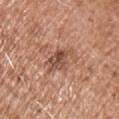Part of a total-body skin-imaging series; this lesion was reviewed on a skin check and was not flagged for biopsy.
About 4.5 mm across.
From the chest.
A 15 mm close-up tile from a total-body photography series done for melanoma screening.
A male subject, aged around 75.
An algorithmic analysis of the crop reported an area of roughly 8 mm² and an outline eccentricity of about 0.85 (0 = round, 1 = elongated). And it measured a mean CIELAB color near L≈51 a*≈22 b*≈29 and roughly 11 lightness units darker than nearby skin. The software also gave a border-irregularity rating of about 4.5/10, a color-variation rating of about 5/10, and peripheral color asymmetry of about 2.